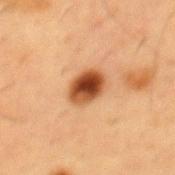Impression:
Captured during whole-body skin photography for melanoma surveillance; the lesion was not biopsied.
Image and clinical context:
On the front of the torso. A 15 mm crop from a total-body photograph taken for skin-cancer surveillance. The lesion's longest dimension is about 4 mm. A male subject, aged 53–57. Imaged with cross-polarized lighting.